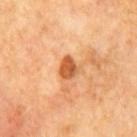Clinical impression: Captured during whole-body skin photography for melanoma surveillance; the lesion was not biopsied. Image and clinical context: A roughly 15 mm field-of-view crop from a total-body skin photograph. Imaged with cross-polarized lighting. The lesion is located on the chest. A male subject, aged around 65. The recorded lesion diameter is about 2.5 mm.